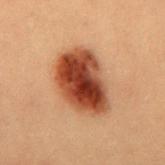Recorded during total-body skin imaging; not selected for excision or biopsy.